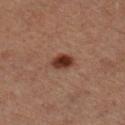Imaged during a routine full-body skin examination; the lesion was not biopsied and no histopathology is available.
The total-body-photography lesion software estimated a footprint of about 5 mm², a shape eccentricity near 0.6, and a symmetry-axis asymmetry near 0.15. And it measured a border-irregularity rating of about 1.5/10 and internal color variation of about 3 on a 0–10 scale. The software also gave an automated nevus-likeness rating near 100 out of 100 and a detector confidence of about 100 out of 100 that the crop contains a lesion.
Imaged with cross-polarized lighting.
From the right lower leg.
The recorded lesion diameter is about 2.5 mm.
Cropped from a whole-body photographic skin survey; the tile spans about 15 mm.
The patient is a male aged 58 to 62.